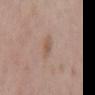Clinical impression: Imaged during a routine full-body skin examination; the lesion was not biopsied and no histopathology is available. Context: Imaged with white-light lighting. A male patient approximately 70 years of age. Longest diameter approximately 3.5 mm. Automated image analysis of the tile measured a lesion color around L≈56 a*≈17 b*≈28 in CIELAB and a lesion-to-skin contrast of about 5.5 (normalized; higher = more distinct). And it measured border irregularity of about 2.5 on a 0–10 scale, a within-lesion color-variation index near 2/10, and a peripheral color-asymmetry measure near 0.5. The analysis additionally found a classifier nevus-likeness of about 0/100 and a lesion-detection confidence of about 100/100. The lesion is on the mid back. A region of skin cropped from a whole-body photographic capture, roughly 15 mm wide.